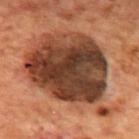The lesion is located on the back. Longest diameter approximately 12 mm. A roughly 15 mm field-of-view crop from a total-body skin photograph. The patient is a male aged 68–72. Captured under cross-polarized illumination.